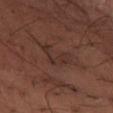{"biopsy_status": "not biopsied; imaged during a skin examination", "site": "chest", "patient": {"sex": "female", "age_approx": 65}, "lighting": "white-light", "automated_metrics": {"nevus_likeness_0_100": 0, "lesion_detection_confidence_0_100": 55}, "lesion_size": {"long_diameter_mm_approx": 4.0}, "image": {"source": "total-body photography crop", "field_of_view_mm": 15}}